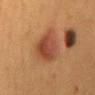workup: imaged on a skin check; not biopsied
TBP lesion metrics: an area of roughly 12 mm², an eccentricity of roughly 0.7, and a shape-asymmetry score of about 0.2 (0 = symmetric); a lesion color around L≈37 a*≈21 b*≈28 in CIELAB, about 9 CIELAB-L* units darker than the surrounding skin, and a normalized lesion–skin contrast near 8
anatomic site: the mid back
image: total-body-photography crop, ~15 mm field of view
subject: female, aged around 50